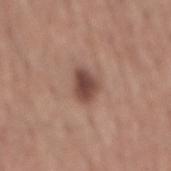Captured during whole-body skin photography for melanoma surveillance; the lesion was not biopsied.
The lesion is on the mid back.
A 15 mm close-up tile from a total-body photography series done for melanoma screening.
The subject is a male about 55 years old.